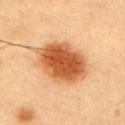Q: Was this lesion biopsied?
A: catalogued during a skin exam; not biopsied
Q: Patient demographics?
A: male, in their mid- to late 50s
Q: How large is the lesion?
A: about 7 mm
Q: What lighting was used for the tile?
A: cross-polarized illumination
Q: What kind of image is this?
A: total-body-photography crop, ~15 mm field of view
Q: Where on the body is the lesion?
A: the arm